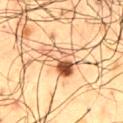Clinical impression:
Captured during whole-body skin photography for melanoma surveillance; the lesion was not biopsied.
Context:
An algorithmic analysis of the crop reported a lesion area of about 9.5 mm², a shape eccentricity near 0.7, and a symmetry-axis asymmetry near 0.55. It also reported a border-irregularity index near 6.5/10, a within-lesion color-variation index near 10/10, and a peripheral color-asymmetry measure near 7.5. It also reported an automated nevus-likeness rating near 95 out of 100 and a lesion-detection confidence of about 100/100. A male subject, aged 58–62. A 15 mm crop from a total-body photograph taken for skin-cancer surveillance. On the mid back. Approximately 4.5 mm at its widest.